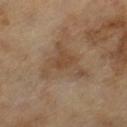The lesion was tiled from a total-body skin photograph and was not biopsied.
Automated tile analysis of the lesion measured a lesion area of about 13 mm², an eccentricity of roughly 0.65, and two-axis asymmetry of about 0.65. The analysis additionally found roughly 7 lightness units darker than nearby skin. The software also gave a classifier nevus-likeness of about 0/100 and lesion-presence confidence of about 100/100.
The tile uses cross-polarized illumination.
A male subject, aged 63–67.
A 15 mm close-up extracted from a 3D total-body photography capture.